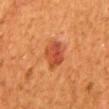Impression:
The lesion was tiled from a total-body skin photograph and was not biopsied.
Context:
About 3.5 mm across. This is a cross-polarized tile. The patient is a male about 45 years old. The lesion is on the mid back. A 15 mm close-up tile from a total-body photography series done for melanoma screening.